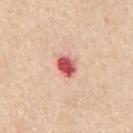Case summary:
– image: ~15 mm tile from a whole-body skin photo
– anatomic site: the back
– patient: male, in their 60s